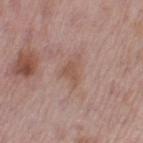Context: Measured at roughly 3.5 mm in maximum diameter. Automated image analysis of the tile measured a lesion area of about 4 mm² and a symmetry-axis asymmetry near 0.6. The software also gave a lesion–skin lightness drop of about 7 and a normalized lesion–skin contrast near 6. The software also gave a border-irregularity rating of about 6.5/10, a color-variation rating of about 1/10, and peripheral color asymmetry of about 0.5. Located on the leg. Cropped from a whole-body photographic skin survey; the tile spans about 15 mm. This is a white-light tile. A female patient, roughly 50 years of age.